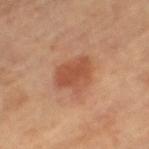{"site": "leg", "patient": {"sex": "female", "age_approx": 70}, "lighting": "cross-polarized", "lesion_size": {"long_diameter_mm_approx": 4.5}, "image": {"source": "total-body photography crop", "field_of_view_mm": 15}}